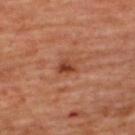follow-up = catalogued during a skin exam; not biopsied
illumination = cross-polarized illumination
acquisition = total-body-photography crop, ~15 mm field of view
diameter = ≈2.5 mm
patient = female, approximately 45 years of age
location = the back